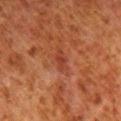Cropped from a whole-body photographic skin survey; the tile spans about 15 mm.
Captured under cross-polarized illumination.
The patient is a male roughly 80 years of age.
The lesion is on the right lower leg.